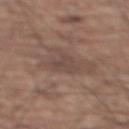This lesion was catalogued during total-body skin photography and was not selected for biopsy. A roughly 15 mm field-of-view crop from a total-body skin photograph. The lesion is on the upper back. This is a white-light tile. The patient is a male aged 73 to 77.